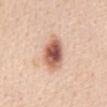The lesion was tiled from a total-body skin photograph and was not biopsied. Captured under white-light illumination. Cropped from a total-body skin-imaging series; the visible field is about 15 mm. The recorded lesion diameter is about 5 mm. The lesion is located on the chest. The subject is a female roughly 45 years of age.